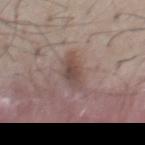workup=total-body-photography surveillance lesion; no biopsy.